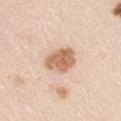Imaged during a routine full-body skin examination; the lesion was not biopsied and no histopathology is available. Longest diameter approximately 4 mm. This is a white-light tile. A roughly 15 mm field-of-view crop from a total-body skin photograph. A male subject about 45 years old. Automated image analysis of the tile measured a lesion area of about 10 mm², an outline eccentricity of about 0.6 (0 = round, 1 = elongated), and a shape-asymmetry score of about 0.2 (0 = symmetric). And it measured a mean CIELAB color near L≈65 a*≈20 b*≈33, about 14 CIELAB-L* units darker than the surrounding skin, and a lesion-to-skin contrast of about 9 (normalized; higher = more distinct). It also reported a color-variation rating of about 3.5/10. The lesion is located on the left upper arm.